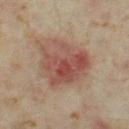Q: Was a biopsy performed?
A: total-body-photography surveillance lesion; no biopsy
Q: Illumination type?
A: cross-polarized
Q: How was this image acquired?
A: ~15 mm tile from a whole-body skin photo
Q: How large is the lesion?
A: ≈6.5 mm
Q: What are the patient's age and sex?
A: female, about 35 years old
Q: Automated lesion metrics?
A: about 10 CIELAB-L* units darker than the surrounding skin; an automated nevus-likeness rating near 10 out of 100 and lesion-presence confidence of about 100/100
Q: Lesion location?
A: the left thigh